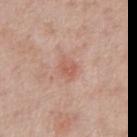biopsy status: no biopsy performed (imaged during a skin exam); anatomic site: the chest; image source: ~15 mm tile from a whole-body skin photo; patient: male, aged 58 to 62; diameter: ≈3 mm.